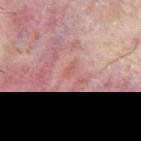Q: Is there a histopathology result?
A: imaged on a skin check; not biopsied
Q: What are the patient's age and sex?
A: male, in their 60s
Q: How was this image acquired?
A: ~15 mm tile from a whole-body skin photo
Q: What lighting was used for the tile?
A: cross-polarized illumination
Q: What is the anatomic site?
A: the abdomen